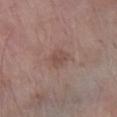| feature | finding |
|---|---|
| patient | female, aged around 75 |
| lighting | white-light illumination |
| body site | the leg |
| image | total-body-photography crop, ~15 mm field of view |